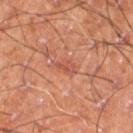Clinical impression: Recorded during total-body skin imaging; not selected for excision or biopsy. Context: On the right thigh. Automated tile analysis of the lesion measured roughly 7 lightness units darker than nearby skin. A roughly 15 mm field-of-view crop from a total-body skin photograph. The subject is a male about 60 years old. Imaged with cross-polarized lighting. Approximately 2.5 mm at its widest.